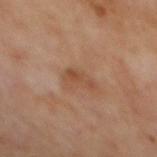{"biopsy_status": "not biopsied; imaged during a skin examination", "lesion_size": {"long_diameter_mm_approx": 3.0}, "image": {"source": "total-body photography crop", "field_of_view_mm": 15}, "lighting": "cross-polarized", "automated_metrics": {"area_mm2_approx": 3.0, "eccentricity": 0.9, "border_irregularity_0_10": 7.0, "color_variation_0_10": 1.0, "peripheral_color_asymmetry": 0.0, "nevus_likeness_0_100": 0, "lesion_detection_confidence_0_100": 100}, "patient": {"sex": "male", "age_approx": 70}, "site": "mid back"}